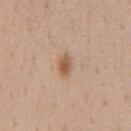follow-up=imaged on a skin check; not biopsied
body site=the chest
acquisition=15 mm crop, total-body photography
illumination=white-light
size=about 2.5 mm
patient=male, approximately 55 years of age